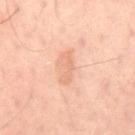follow-up — total-body-photography surveillance lesion; no biopsy
anatomic site — the left leg
acquisition — total-body-photography crop, ~15 mm field of view
diameter — ≈3.5 mm
illumination — cross-polarized
patient — male, about 50 years old
image-analysis metrics — border irregularity of about 4.5 on a 0–10 scale and a peripheral color-asymmetry measure near 0.5; a lesion-detection confidence of about 100/100